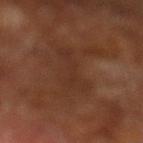Findings:
* notes: catalogued during a skin exam; not biopsied
* subject: male, approximately 65 years of age
* body site: the left lower leg
* illumination: cross-polarized illumination
* diameter: ≈7 mm
* acquisition: total-body-photography crop, ~15 mm field of view